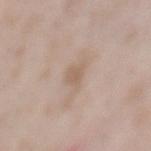Recorded during total-body skin imaging; not selected for excision or biopsy. A male patient, aged approximately 55. Captured under white-light illumination. About 2.5 mm across. On the mid back. A roughly 15 mm field-of-view crop from a total-body skin photograph.